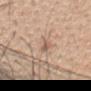Notes:
- follow-up · total-body-photography surveillance lesion; no biopsy
- tile lighting · white-light
- acquisition · 15 mm crop, total-body photography
- body site · the head or neck
- automated metrics · an outline eccentricity of about 0.9 (0 = round, 1 = elongated) and a symmetry-axis asymmetry near 0.3; an average lesion color of about L≈60 a*≈16 b*≈29 (CIELAB), roughly 8 lightness units darker than nearby skin, and a normalized border contrast of about 5.5; a nevus-likeness score of about 0/100 and a detector confidence of about 90 out of 100 that the crop contains a lesion
- subject · female, in their 50s
- size · ~3 mm (longest diameter)